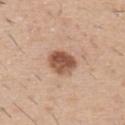* workup: catalogued during a skin exam; not biopsied
* image source: 15 mm crop, total-body photography
* size: ≈3.5 mm
* automated lesion analysis: an area of roughly 8 mm², an outline eccentricity of about 0.6 (0 = round, 1 = elongated), and a shape-asymmetry score of about 0.2 (0 = symmetric); an average lesion color of about L≈53 a*≈22 b*≈30 (CIELAB) and a lesion-to-skin contrast of about 10.5 (normalized; higher = more distinct); a border-irregularity index near 1.5/10; an automated nevus-likeness rating near 95 out of 100 and a lesion-detection confidence of about 100/100
* patient: male, aged approximately 60
* location: the right upper arm
* illumination: white-light illumination